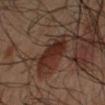Notes:
* notes: imaged on a skin check; not biopsied
* location: the left arm
* image source: total-body-photography crop, ~15 mm field of view
* patient: male, aged around 50
* diameter: about 4.5 mm